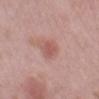Recorded during total-body skin imaging; not selected for excision or biopsy. The tile uses white-light illumination. The patient is a male in their mid- to late 50s. On the arm. Cropped from a whole-body photographic skin survey; the tile spans about 15 mm. About 2.5 mm across.